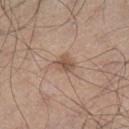The lesion was photographed on a routine skin check and not biopsied; there is no pathology result.
A male subject, about 45 years old.
The total-body-photography lesion software estimated an area of roughly 5 mm², an outline eccentricity of about 0.6 (0 = round, 1 = elongated), and two-axis asymmetry of about 0.25. The software also gave roughly 10 lightness units darker than nearby skin and a lesion-to-skin contrast of about 7 (normalized; higher = more distinct). And it measured a border-irregularity index near 2.5/10 and internal color variation of about 2.5 on a 0–10 scale. And it measured a classifier nevus-likeness of about 40/100.
This image is a 15 mm lesion crop taken from a total-body photograph.
Imaged with white-light lighting.
On the left lower leg.
The recorded lesion diameter is about 3 mm.